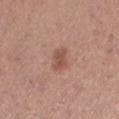Context: About 3 mm across. Cropped from a total-body skin-imaging series; the visible field is about 15 mm. On the left lower leg. Imaged with white-light lighting. The patient is a female aged approximately 30.